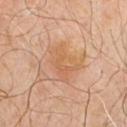<record>
<biopsy_status>not biopsied; imaged during a skin examination</biopsy_status>
<lesion_size>
  <long_diameter_mm_approx>4.5</long_diameter_mm_approx>
</lesion_size>
<lighting>cross-polarized</lighting>
<patient>
  <sex>male</sex>
  <age_approx>55</age_approx>
</patient>
<site>chest</site>
<automated_metrics>
  <cielab_L>59</cielab_L>
  <cielab_a>21</cielab_a>
  <cielab_b>35</cielab_b>
  <vs_skin_darker_L>6.0</vs_skin_darker_L>
  <vs_skin_contrast_norm>5.5</vs_skin_contrast_norm>
</automated_metrics>
<image>
  <source>total-body photography crop</source>
  <field_of_view_mm>15</field_of_view_mm>
</image>
</record>